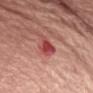{
  "patient": {
    "sex": "female",
    "age_approx": 65
  },
  "image": {
    "source": "total-body photography crop",
    "field_of_view_mm": 15
  },
  "site": "left upper arm"
}